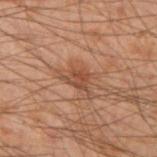- follow-up — imaged on a skin check; not biopsied
- body site — the left arm
- acquisition — total-body-photography crop, ~15 mm field of view
- patient — male, roughly 50 years of age